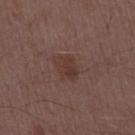biopsy status: catalogued during a skin exam; not biopsied
illumination: white-light
anatomic site: the mid back
image: ~15 mm tile from a whole-body skin photo
lesion diameter: about 3.5 mm
subject: male, aged around 50